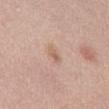Imaged during a routine full-body skin examination; the lesion was not biopsied and no histopathology is available. Automated image analysis of the tile measured an area of roughly 3 mm², an outline eccentricity of about 0.9 (0 = round, 1 = elongated), and a shape-asymmetry score of about 0.3 (0 = symmetric). And it measured an average lesion color of about L≈62 a*≈19 b*≈29 (CIELAB), about 7 CIELAB-L* units darker than the surrounding skin, and a lesion-to-skin contrast of about 5.5 (normalized; higher = more distinct). It also reported a border-irregularity rating of about 3/10, a within-lesion color-variation index near 3.5/10, and a peripheral color-asymmetry measure near 1.5. About 3 mm across. A lesion tile, about 15 mm wide, cut from a 3D total-body photograph. On the chest. The tile uses white-light illumination. The subject is a female about 65 years old.